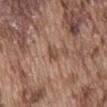Assessment: No biopsy was performed on this lesion — it was imaged during a full skin examination and was not determined to be concerning. Clinical summary: Located on the front of the torso. The lesion's longest dimension is about 2.5 mm. A 15 mm close-up extracted from a 3D total-body photography capture. The tile uses white-light illumination. A male patient, aged approximately 75.